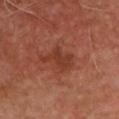<lesion>
<biopsy_status>not biopsied; imaged during a skin examination</biopsy_status>
<patient>
  <sex>male</sex>
  <age_approx>50</age_approx>
</patient>
<lighting>cross-polarized</lighting>
<site>chest</site>
<image>
  <source>total-body photography crop</source>
  <field_of_view_mm>15</field_of_view_mm>
</image>
</lesion>